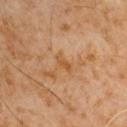Findings:
– follow-up: catalogued during a skin exam; not biopsied
– acquisition: ~15 mm crop, total-body skin-cancer survey
– automated lesion analysis: an area of roughly 3 mm² and a symmetry-axis asymmetry near 0.4; an average lesion color of about L≈50 a*≈20 b*≈38 (CIELAB), a lesion–skin lightness drop of about 6, and a normalized lesion–skin contrast near 6; internal color variation of about 0.5 on a 0–10 scale
– body site: the arm
– size: about 3 mm
– subject: male, aged around 60
– tile lighting: cross-polarized